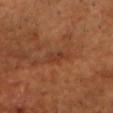* workup: catalogued during a skin exam; not biopsied
* patient: male, in their 60s
* image: total-body-photography crop, ~15 mm field of view
* site: the chest
* diameter: about 3 mm
* TBP lesion metrics: a lesion area of about 3.5 mm²; a lesion color around L≈36 a*≈24 b*≈31 in CIELAB, roughly 6 lightness units darker than nearby skin, and a lesion-to-skin contrast of about 5.5 (normalized; higher = more distinct)